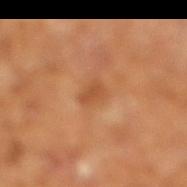Q: Is there a histopathology result?
A: no biopsy performed (imaged during a skin exam)
Q: Illumination type?
A: cross-polarized illumination
Q: Patient demographics?
A: male, aged around 65
Q: What kind of image is this?
A: 15 mm crop, total-body photography
Q: What did automated image analysis measure?
A: a border-irregularity rating of about 2.5/10 and a peripheral color-asymmetry measure near 0; a classifier nevus-likeness of about 0/100